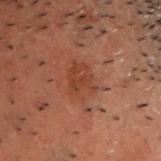This lesion was catalogued during total-body skin photography and was not selected for biopsy.
The subject is a male about 50 years old.
Located on the chest.
Imaged with cross-polarized lighting.
A close-up tile cropped from a whole-body skin photograph, about 15 mm across.